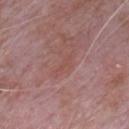Q: Illumination type?
A: white-light illumination
Q: What is the anatomic site?
A: the chest
Q: How was this image acquired?
A: ~15 mm crop, total-body skin-cancer survey
Q: Automated lesion metrics?
A: an area of roughly 3 mm², an outline eccentricity of about 0.95 (0 = round, 1 = elongated), and a symmetry-axis asymmetry near 0.35
Q: Patient demographics?
A: male, approximately 65 years of age
Q: How large is the lesion?
A: about 3 mm